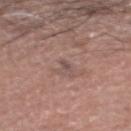Clinical impression: The lesion was photographed on a routine skin check and not biopsied; there is no pathology result. Clinical summary: A 15 mm crop from a total-body photograph taken for skin-cancer surveillance. Located on the head or neck. The patient is a male approximately 45 years of age. Approximately 1.5 mm at its widest. Imaged with white-light lighting. The lesion-visualizer software estimated a color-variation rating of about 0/10 and radial color variation of about 0. The analysis additionally found an automated nevus-likeness rating near 0 out of 100.